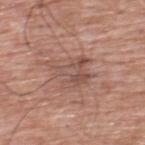The lesion was tiled from a total-body skin photograph and was not biopsied. On the upper back. A region of skin cropped from a whole-body photographic capture, roughly 15 mm wide. A male subject, in their 60s.